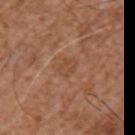| field | value |
|---|---|
| notes | imaged on a skin check; not biopsied |
| acquisition | ~15 mm tile from a whole-body skin photo |
| subject | male, in their mid- to late 70s |
| TBP lesion metrics | an area of roughly 3.5 mm², a shape eccentricity near 0.75, and a shape-asymmetry score of about 0.25 (0 = symmetric); an average lesion color of about L≈47 a*≈21 b*≈32 (CIELAB), a lesion–skin lightness drop of about 6, and a normalized lesion–skin contrast near 4.5; border irregularity of about 3 on a 0–10 scale, a color-variation rating of about 1.5/10, and peripheral color asymmetry of about 0.5 |
| site | the left upper arm |